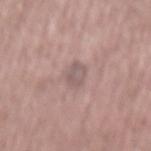<case>
  <biopsy_status>not biopsied; imaged during a skin examination</biopsy_status>
  <image>
    <source>total-body photography crop</source>
    <field_of_view_mm>15</field_of_view_mm>
  </image>
  <lighting>white-light</lighting>
  <patient>
    <sex>male</sex>
    <age_approx>60</age_approx>
  </patient>
  <lesion_size>
    <long_diameter_mm_approx>3.5</long_diameter_mm_approx>
  </lesion_size>
  <site>mid back</site>
</case>